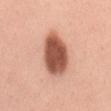Assessment:
Recorded during total-body skin imaging; not selected for excision or biopsy.
Background:
This image is a 15 mm lesion crop taken from a total-body photograph. The total-body-photography lesion software estimated a footprint of about 16 mm², an eccentricity of roughly 0.8, and two-axis asymmetry of about 0.1. And it measured a mean CIELAB color near L≈54 a*≈26 b*≈31, about 20 CIELAB-L* units darker than the surrounding skin, and a lesion-to-skin contrast of about 12.5 (normalized; higher = more distinct). Longest diameter approximately 6 mm. Captured under white-light illumination. A female subject aged 33–37. The lesion is located on the mid back.